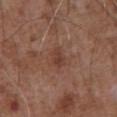subject — male, aged 73 to 77
location — the abdomen
lighting — white-light
lesion size — ≈2.5 mm
acquisition — ~15 mm tile from a whole-body skin photo
TBP lesion metrics — an outline eccentricity of about 0.7 (0 = round, 1 = elongated) and a symmetry-axis asymmetry near 0.3; a border-irregularity index near 2.5/10, a within-lesion color-variation index near 2.5/10, and peripheral color asymmetry of about 1; a nevus-likeness score of about 5/100 and lesion-presence confidence of about 100/100